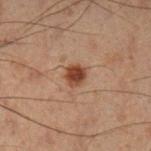Q: Was this lesion biopsied?
A: catalogued during a skin exam; not biopsied
Q: What did automated image analysis measure?
A: a lesion color around L≈33 a*≈18 b*≈25 in CIELAB, a lesion–skin lightness drop of about 11, and a lesion-to-skin contrast of about 10.5 (normalized; higher = more distinct); a nevus-likeness score of about 100/100 and a detector confidence of about 100 out of 100 that the crop contains a lesion
Q: What is the imaging modality?
A: ~15 mm crop, total-body skin-cancer survey
Q: What is the lesion's diameter?
A: ~2.5 mm (longest diameter)
Q: Lesion location?
A: the left lower leg
Q: What are the patient's age and sex?
A: male, aged 48–52
Q: Illumination type?
A: cross-polarized